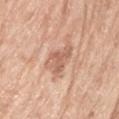<case>
  <biopsy_status>not biopsied; imaged during a skin examination</biopsy_status>
  <image>
    <source>total-body photography crop</source>
    <field_of_view_mm>15</field_of_view_mm>
  </image>
  <lesion_size>
    <long_diameter_mm_approx>4.0</long_diameter_mm_approx>
  </lesion_size>
  <site>left upper arm</site>
  <automated_metrics>
    <area_mm2_approx>8.0</area_mm2_approx>
    <eccentricity>0.65</eccentricity>
    <shape_asymmetry>0.4</shape_asymmetry>
    <border_irregularity_0_10>4.5</border_irregularity_0_10>
    <peripheral_color_asymmetry>1.0</peripheral_color_asymmetry>
  </automated_metrics>
  <patient>
    <sex>female</sex>
    <age_approx>75</age_approx>
  </patient>
</case>